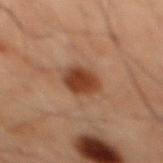This lesion was catalogued during total-body skin photography and was not selected for biopsy. On the mid back. Imaged with cross-polarized lighting. A male patient, aged 58 to 62. The total-body-photography lesion software estimated a lesion area of about 8 mm², an eccentricity of roughly 0.45, and a shape-asymmetry score of about 0.1 (0 = symmetric). The software also gave an average lesion color of about L≈31 a*≈19 b*≈26 (CIELAB) and a normalized lesion–skin contrast near 10.5. It also reported a nevus-likeness score of about 100/100. A close-up tile cropped from a whole-body skin photograph, about 15 mm across.